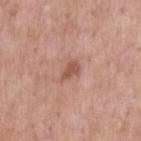Captured during whole-body skin photography for melanoma surveillance; the lesion was not biopsied. This is a white-light tile. The lesion is located on the upper back. The subject is a male in their mid- to late 50s. About 3 mm across. A roughly 15 mm field-of-view crop from a total-body skin photograph.